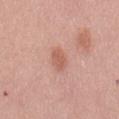Clinical impression: Captured during whole-body skin photography for melanoma surveillance; the lesion was not biopsied. Acquisition and patient details: A lesion tile, about 15 mm wide, cut from a 3D total-body photograph. A female subject, in their mid-60s. The tile uses white-light illumination. The lesion is located on the mid back. The lesion's longest dimension is about 3 mm.